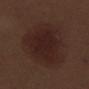Captured during whole-body skin photography for melanoma surveillance; the lesion was not biopsied. The patient is a male in their 70s. The tile uses white-light illumination. A roughly 15 mm field-of-view crop from a total-body skin photograph. The recorded lesion diameter is about 8 mm. The lesion is located on the left thigh. An algorithmic analysis of the crop reported a mean CIELAB color near L≈22 a*≈17 b*≈19, roughly 6 lightness units darker than nearby skin, and a normalized lesion–skin contrast near 7.5. It also reported a border-irregularity index near 3/10, a within-lesion color-variation index near 3/10, and radial color variation of about 1.